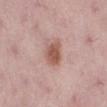<lesion>
  <biopsy_status>not biopsied; imaged during a skin examination</biopsy_status>
  <lesion_size>
    <long_diameter_mm_approx>3.5</long_diameter_mm_approx>
  </lesion_size>
  <site>right lower leg</site>
  <patient>
    <sex>female</sex>
    <age_approx>50</age_approx>
  </patient>
  <image>
    <source>total-body photography crop</source>
    <field_of_view_mm>15</field_of_view_mm>
  </image>
  <automated_metrics>
    <area_mm2_approx>7.0</area_mm2_approx>
    <eccentricity>0.6</eccentricity>
    <border_irregularity_0_10>2.0</border_irregularity_0_10>
    <color_variation_0_10>3.0</color_variation_0_10>
    <peripheral_color_asymmetry>1.0</peripheral_color_asymmetry>
  </automated_metrics>
</lesion>